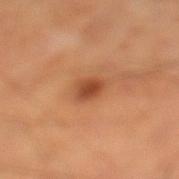| field | value |
|---|---|
| workup | catalogued during a skin exam; not biopsied |
| acquisition | ~15 mm crop, total-body skin-cancer survey |
| site | the left lower leg |
| tile lighting | cross-polarized |
| subject | male, approximately 60 years of age |